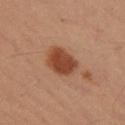Case summary:
– biopsy status: no biopsy performed (imaged during a skin exam)
– body site: the left thigh
– diameter: about 4 mm
– acquisition: ~15 mm tile from a whole-body skin photo
– lighting: cross-polarized
– subject: male, about 40 years old